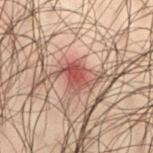follow-up = imaged on a skin check; not biopsied | subject = male, in their 50s | body site = the right thigh | size = ≈4 mm | illumination = cross-polarized | acquisition = ~15 mm tile from a whole-body skin photo.